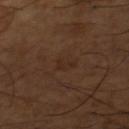follow-up — imaged on a skin check; not biopsied | lighting — cross-polarized | location — the left thigh | image — ~15 mm tile from a whole-body skin photo | patient — male, roughly 65 years of age | lesion diameter — ≈2.5 mm.